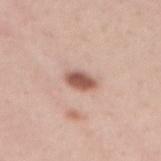Recorded during total-body skin imaging; not selected for excision or biopsy.
From the mid back.
About 3 mm across.
The tile uses white-light illumination.
A female subject, aged approximately 30.
Cropped from a whole-body photographic skin survey; the tile spans about 15 mm.